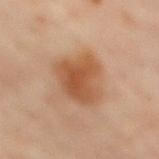Part of a total-body skin-imaging series; this lesion was reviewed on a skin check and was not flagged for biopsy.
The lesion is on the mid back.
A male subject, aged 58–62.
Imaged with cross-polarized lighting.
Approximately 5.5 mm at its widest.
A 15 mm close-up tile from a total-body photography series done for melanoma screening.